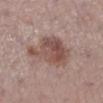{"biopsy_status": "not biopsied; imaged during a skin examination", "automated_metrics": {"nevus_likeness_0_100": 40, "lesion_detection_confidence_0_100": 100}, "patient": {"sex": "female", "age_approx": 55}, "site": "left lower leg", "image": {"source": "total-body photography crop", "field_of_view_mm": 15}}